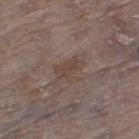{"biopsy_status": "not biopsied; imaged during a skin examination", "image": {"source": "total-body photography crop", "field_of_view_mm": 15}, "automated_metrics": {"cielab_L": 44, "cielab_a": 14, "cielab_b": 21, "vs_skin_contrast_norm": 5.5, "border_irregularity_0_10": 4.5, "peripheral_color_asymmetry": 0.5, "lesion_detection_confidence_0_100": 100}, "lesion_size": {"long_diameter_mm_approx": 4.0}, "site": "left thigh", "lighting": "white-light", "patient": {"sex": "female", "age_approx": "85+"}}